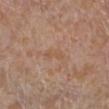Impression:
Part of a total-body skin-imaging series; this lesion was reviewed on a skin check and was not flagged for biopsy.
Acquisition and patient details:
A region of skin cropped from a whole-body photographic capture, roughly 15 mm wide. The lesion is on the right lower leg. The patient is a female in their mid- to late 50s.